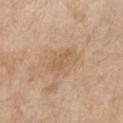Clinical impression: Part of a total-body skin-imaging series; this lesion was reviewed on a skin check and was not flagged for biopsy. Background: The tile uses white-light illumination. A close-up tile cropped from a whole-body skin photograph, about 15 mm across. Located on the chest. A female patient, about 65 years old. About 3.5 mm across.